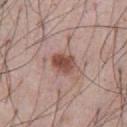| feature | finding |
|---|---|
| follow-up | catalogued during a skin exam; not biopsied |
| lesion size | ~3 mm (longest diameter) |
| location | the abdomen |
| image-analysis metrics | a lesion color around L≈49 a*≈22 b*≈25 in CIELAB and a normalized border contrast of about 9.5; a border-irregularity index near 1.5/10, internal color variation of about 4 on a 0–10 scale, and a peripheral color-asymmetry measure near 1.5; a nevus-likeness score of about 90/100 and a lesion-detection confidence of about 100/100 |
| tile lighting | white-light illumination |
| imaging modality | 15 mm crop, total-body photography |
| patient | male, in their mid- to late 50s |